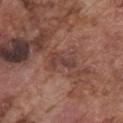The lesion was tiled from a total-body skin photograph and was not biopsied. About 3.5 mm across. The lesion is located on the front of the torso. The tile uses white-light illumination. A male patient roughly 75 years of age. A close-up tile cropped from a whole-body skin photograph, about 15 mm across.